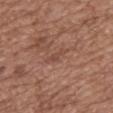Context: This is a white-light tile. From the mid back. The patient is a female aged 73–77. The total-body-photography lesion software estimated an average lesion color of about L≈47 a*≈22 b*≈27 (CIELAB). And it measured a border-irregularity index near 3.5/10 and a peripheral color-asymmetry measure near 0. It also reported a nevus-likeness score of about 0/100. Measured at roughly 2.5 mm in maximum diameter. Cropped from a total-body skin-imaging series; the visible field is about 15 mm.